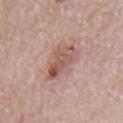notes: no biopsy performed (imaged during a skin exam) | image source: 15 mm crop, total-body photography | tile lighting: white-light illumination | automated lesion analysis: an average lesion color of about L≈54 a*≈21 b*≈24 (CIELAB) and about 10 CIELAB-L* units darker than the surrounding skin; a color-variation rating of about 8/10 and a peripheral color-asymmetry measure near 3 | location: the mid back | size: about 4 mm | patient: male, aged 68–72.